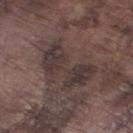notes = catalogued during a skin exam; not biopsied